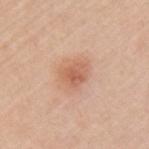This lesion was catalogued during total-body skin photography and was not selected for biopsy.
From the arm.
A male patient aged 58–62.
A 15 mm close-up extracted from a 3D total-body photography capture.